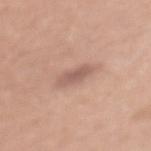The lesion was photographed on a routine skin check and not biopsied; there is no pathology result. Imaged with white-light lighting. A female subject aged around 50. The recorded lesion diameter is about 3 mm. The lesion is on the mid back. A roughly 15 mm field-of-view crop from a total-body skin photograph.